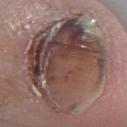No biopsy was performed on this lesion — it was imaged during a full skin examination and was not determined to be concerning.
Longest diameter approximately 12 mm.
A lesion tile, about 15 mm wide, cut from a 3D total-body photograph.
The patient is a male in their mid-70s.
The tile uses white-light illumination.
The lesion is on the leg.